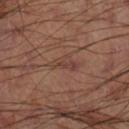Assessment: Captured during whole-body skin photography for melanoma surveillance; the lesion was not biopsied. Context: Cropped from a whole-body photographic skin survey; the tile spans about 15 mm. Approximately 3.5 mm at its widest. Captured under cross-polarized illumination. The lesion is located on the right lower leg.